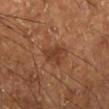No biopsy was performed on this lesion — it was imaged during a full skin examination and was not determined to be concerning. A male patient aged around 65. The recorded lesion diameter is about 3.5 mm. This is a cross-polarized tile. Cropped from a whole-body photographic skin survey; the tile spans about 15 mm. The total-body-photography lesion software estimated an area of roughly 5 mm² and a shape-asymmetry score of about 0.4 (0 = symmetric). The software also gave an automated nevus-likeness rating near 5 out of 100 and lesion-presence confidence of about 100/100. Located on the right lower leg.